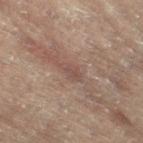notes — catalogued during a skin exam; not biopsied
subject — female, aged 78–82
anatomic site — the left leg
image source — ~15 mm crop, total-body skin-cancer survey